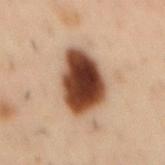Recorded during total-body skin imaging; not selected for excision or biopsy. Longest diameter approximately 7 mm. A 15 mm crop from a total-body photograph taken for skin-cancer surveillance. Located on the mid back. This is a cross-polarized tile. A male subject, aged around 55.